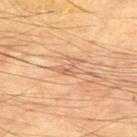<tbp_lesion>
<biopsy_status>not biopsied; imaged during a skin examination</biopsy_status>
<lighting>cross-polarized</lighting>
<site>upper back</site>
<image>
  <source>total-body photography crop</source>
  <field_of_view_mm>15</field_of_view_mm>
</image>
<patient>
  <sex>male</sex>
  <age_approx>75</age_approx>
</patient>
</tbp_lesion>